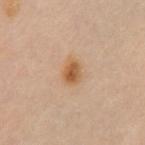Imaged during a routine full-body skin examination; the lesion was not biopsied and no histopathology is available.
Longest diameter approximately 3 mm.
A region of skin cropped from a whole-body photographic capture, roughly 15 mm wide.
The lesion-visualizer software estimated a lesion color around L≈59 a*≈21 b*≈38 in CIELAB, roughly 11 lightness units darker than nearby skin, and a lesion-to-skin contrast of about 9 (normalized; higher = more distinct).
This is a cross-polarized tile.
From the chest.
The patient is a female roughly 60 years of age.